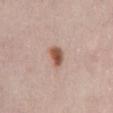biopsy_status: not biopsied; imaged during a skin examination
image:
  source: total-body photography crop
  field_of_view_mm: 15
site: abdomen
automated_metrics:
  cielab_L: 54
  cielab_a: 21
  cielab_b: 29
  vs_skin_darker_L: 15.0
  vs_skin_contrast_norm: 10.5
  border_irregularity_0_10: 2.0
  color_variation_0_10: 5.0
  peripheral_color_asymmetry: 1.5
  nevus_likeness_0_100: 100
  lesion_detection_confidence_0_100: 100
lighting: white-light
patient:
  sex: female
  age_approx: 65
lesion_size:
  long_diameter_mm_approx: 2.5